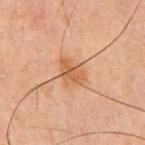Imaged during a routine full-body skin examination; the lesion was not biopsied and no histopathology is available.
Imaged with cross-polarized lighting.
A male patient, about 60 years old.
The lesion is located on the chest.
A 15 mm crop from a total-body photograph taken for skin-cancer surveillance.
The lesion's longest dimension is about 3.5 mm.